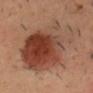* lesion diameter — about 9.5 mm
* subject — male, aged 33 to 37
* tile lighting — cross-polarized
* site — the head or neck
* acquisition — total-body-photography crop, ~15 mm field of view